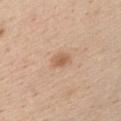| key | value |
|---|---|
| follow-up | catalogued during a skin exam; not biopsied |
| lesion size | ≈2.5 mm |
| image | total-body-photography crop, ~15 mm field of view |
| subject | male, aged 43 to 47 |
| lighting | white-light |
| site | the chest |